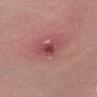| field | value |
|---|---|
| biopsy status | catalogued during a skin exam; not biopsied |
| acquisition | ~15 mm tile from a whole-body skin photo |
| subject | male, aged 58 to 62 |
| TBP lesion metrics | a lesion color around L≈47 a*≈30 b*≈22 in CIELAB and about 10 CIELAB-L* units darker than the surrounding skin; border irregularity of about 3.5 on a 0–10 scale, internal color variation of about 6 on a 0–10 scale, and a peripheral color-asymmetry measure near 1.5 |
| lesion diameter | ~3.5 mm (longest diameter) |
| body site | the left forearm |